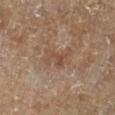Captured during whole-body skin photography for melanoma surveillance; the lesion was not biopsied. Imaged with cross-polarized lighting. The recorded lesion diameter is about 4 mm. A male patient, roughly 85 years of age or older. The total-body-photography lesion software estimated a color-variation rating of about 3/10. It also reported a classifier nevus-likeness of about 0/100 and a detector confidence of about 100 out of 100 that the crop contains a lesion. A region of skin cropped from a whole-body photographic capture, roughly 15 mm wide. Located on the left lower leg.